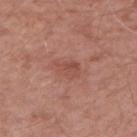This lesion was catalogued during total-body skin photography and was not selected for biopsy.